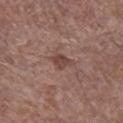workup — no biopsy performed (imaged during a skin exam) | lesion size — ~2.5 mm (longest diameter) | patient — male, aged 68 to 72 | imaging modality — ~15 mm tile from a whole-body skin photo | image-analysis metrics — an area of roughly 3.5 mm² and a symmetry-axis asymmetry near 0.3; a mean CIELAB color near L≈43 a*≈20 b*≈23, roughly 9 lightness units darker than nearby skin, and a normalized border contrast of about 7.5; an automated nevus-likeness rating near 5 out of 100 | site — the right lower leg.